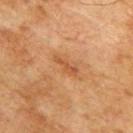Imaged during a routine full-body skin examination; the lesion was not biopsied and no histopathology is available. The lesion-visualizer software estimated a border-irregularity rating of about 4/10, a color-variation rating of about 1/10, and radial color variation of about 0. The subject is a male about 75 years old. From the upper back. Cropped from a total-body skin-imaging series; the visible field is about 15 mm.